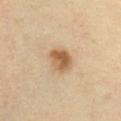notes: no biopsy performed (imaged during a skin exam) | site: the chest | diameter: about 3 mm | tile lighting: cross-polarized | patient: female, roughly 40 years of age | automated metrics: an outline eccentricity of about 0.55 (0 = round, 1 = elongated) and a shape-asymmetry score of about 0.2 (0 = symmetric); a mean CIELAB color near L≈59 a*≈18 b*≈38; a border-irregularity index near 2/10, a color-variation rating of about 5/10, and radial color variation of about 2; a nevus-likeness score of about 95/100 and lesion-presence confidence of about 100/100 | image: ~15 mm crop, total-body skin-cancer survey.